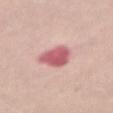notes: catalogued during a skin exam; not biopsied
tile lighting: white-light
lesion size: ~4.5 mm (longest diameter)
image-analysis metrics: a shape eccentricity near 0.75; an average lesion color of about L≈59 a*≈32 b*≈21 (CIELAB) and about 15 CIELAB-L* units darker than the surrounding skin; a border-irregularity index near 2.5/10; a nevus-likeness score of about 10/100 and a lesion-detection confidence of about 100/100
image: total-body-photography crop, ~15 mm field of view
subject: female, aged 53 to 57
body site: the abdomen